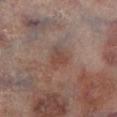Captured during whole-body skin photography for melanoma surveillance; the lesion was not biopsied.
The tile uses cross-polarized illumination.
From the left lower leg.
A 15 mm close-up extracted from a 3D total-body photography capture.
A male patient in their mid-60s.